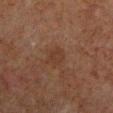{"biopsy_status": "not biopsied; imaged during a skin examination", "lighting": "cross-polarized", "patient": {"sex": "male", "age_approx": 60}, "site": "left upper arm", "image": {"source": "total-body photography crop", "field_of_view_mm": 15}, "lesion_size": {"long_diameter_mm_approx": 2.5}}